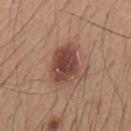Q: Was this lesion biopsied?
A: imaged on a skin check; not biopsied
Q: Where on the body is the lesion?
A: the back
Q: What did automated image analysis measure?
A: an area of roughly 14 mm², an outline eccentricity of about 0.7 (0 = round, 1 = elongated), and a shape-asymmetry score of about 0.25 (0 = symmetric); border irregularity of about 2.5 on a 0–10 scale and a within-lesion color-variation index near 5/10; a classifier nevus-likeness of about 90/100 and a lesion-detection confidence of about 100/100
Q: How was the tile lit?
A: white-light illumination
Q: Patient demographics?
A: male, approximately 20 years of age
Q: What is the imaging modality?
A: total-body-photography crop, ~15 mm field of view
Q: How large is the lesion?
A: ≈5 mm